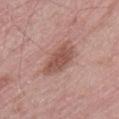Recorded during total-body skin imaging; not selected for excision or biopsy.
A 15 mm close-up tile from a total-body photography series done for melanoma screening.
From the left thigh.
The patient is a male aged approximately 70.